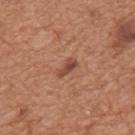Impression: The lesion was tiled from a total-body skin photograph and was not biopsied. Acquisition and patient details: The lesion is on the back. A male patient, aged 63–67. The lesion's longest dimension is about 2.5 mm. The total-body-photography lesion software estimated a lesion–skin lightness drop of about 11 and a lesion-to-skin contrast of about 8 (normalized; higher = more distinct). The software also gave a peripheral color-asymmetry measure near 0. A roughly 15 mm field-of-view crop from a total-body skin photograph. Captured under white-light illumination.